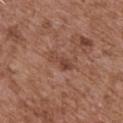workup: imaged on a skin check; not biopsied
patient: male, about 75 years old
body site: the front of the torso
acquisition: ~15 mm tile from a whole-body skin photo